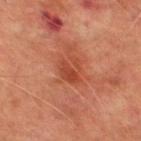Captured during whole-body skin photography for melanoma surveillance; the lesion was not biopsied.
An algorithmic analysis of the crop reported a lesion color around L≈38 a*≈27 b*≈30 in CIELAB and a lesion–skin lightness drop of about 7. And it measured border irregularity of about 3 on a 0–10 scale, a color-variation rating of about 4/10, and a peripheral color-asymmetry measure near 1.5.
Captured under cross-polarized illumination.
A 15 mm crop from a total-body photograph taken for skin-cancer surveillance.
On the left thigh.
About 3.5 mm across.
A male subject aged around 75.